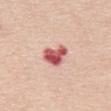Q: Was this lesion biopsied?
A: no biopsy performed (imaged during a skin exam)
Q: What is the anatomic site?
A: the abdomen
Q: What is the imaging modality?
A: 15 mm crop, total-body photography
Q: What did automated image analysis measure?
A: a footprint of about 7 mm², an outline eccentricity of about 0.75 (0 = round, 1 = elongated), and a shape-asymmetry score of about 0.35 (0 = symmetric); an average lesion color of about L≈58 a*≈34 b*≈24 (CIELAB) and a normalized border contrast of about 11.5; border irregularity of about 3.5 on a 0–10 scale, a color-variation rating of about 6.5/10, and a peripheral color-asymmetry measure near 2
Q: Patient demographics?
A: male, roughly 65 years of age
Q: How large is the lesion?
A: ≈3.5 mm